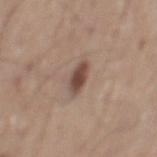Recorded during total-body skin imaging; not selected for excision or biopsy. Imaged with white-light lighting. Approximately 3.5 mm at its widest. A 15 mm crop from a total-body photograph taken for skin-cancer surveillance. The lesion is on the mid back. A male subject in their mid- to late 70s. The total-body-photography lesion software estimated an area of roughly 5 mm², an outline eccentricity of about 0.85 (0 = round, 1 = elongated), and a symmetry-axis asymmetry near 0.2. It also reported a classifier nevus-likeness of about 95/100 and a detector confidence of about 100 out of 100 that the crop contains a lesion.